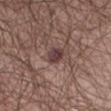follow-up: catalogued during a skin exam; not biopsied
image source: total-body-photography crop, ~15 mm field of view
automated metrics: a footprint of about 4 mm², an eccentricity of roughly 0.65, and a shape-asymmetry score of about 0.25 (0 = symmetric); a lesion color around L≈39 a*≈20 b*≈17 in CIELAB and roughly 11 lightness units darker than nearby skin; border irregularity of about 2 on a 0–10 scale and radial color variation of about 1; an automated nevus-likeness rating near 35 out of 100 and a lesion-detection confidence of about 100/100
lighting: white-light illumination
subject: male, in their mid- to late 80s or older
lesion size: about 2.5 mm
body site: the abdomen